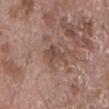The lesion was photographed on a routine skin check and not biopsied; there is no pathology result.